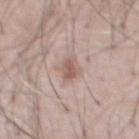workup: no biopsy performed (imaged during a skin exam)
image-analysis metrics: a mean CIELAB color near L≈57 a*≈17 b*≈24, about 10 CIELAB-L* units darker than the surrounding skin, and a lesion-to-skin contrast of about 7 (normalized; higher = more distinct); internal color variation of about 1.5 on a 0–10 scale and a peripheral color-asymmetry measure near 0.5; a classifier nevus-likeness of about 70/100
image: ~15 mm crop, total-body skin-cancer survey
anatomic site: the abdomen
patient: male, aged 63–67
diameter: about 3 mm
lighting: white-light illumination